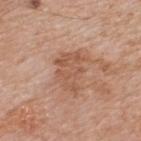<case>
<biopsy_status>not biopsied; imaged during a skin examination</biopsy_status>
<lighting>white-light</lighting>
<site>upper back</site>
<patient>
  <sex>male</sex>
  <age_approx>60</age_approx>
</patient>
<lesion_size>
  <long_diameter_mm_approx>4.5</long_diameter_mm_approx>
</lesion_size>
<automated_metrics>
  <area_mm2_approx>10.0</area_mm2_approx>
  <eccentricity>0.75</eccentricity>
  <shape_asymmetry>0.45</shape_asymmetry>
  <nevus_likeness_0_100>0</nevus_likeness_0_100>
  <lesion_detection_confidence_0_100>100</lesion_detection_confidence_0_100>
</automated_metrics>
<image>
  <source>total-body photography crop</source>
  <field_of_view_mm>15</field_of_view_mm>
</image>
</case>